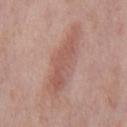{"site": "mid back", "image": {"source": "total-body photography crop", "field_of_view_mm": 15}, "patient": {"sex": "male", "age_approx": 75}, "lesion_size": {"long_diameter_mm_approx": 8.0}}